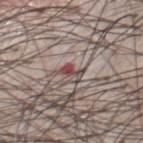Assessment: Part of a total-body skin-imaging series; this lesion was reviewed on a skin check and was not flagged for biopsy. Background: Measured at roughly 2.5 mm in maximum diameter. The lesion is located on the chest. A lesion tile, about 15 mm wide, cut from a 3D total-body photograph. The tile uses white-light illumination. The patient is a male in their 60s. An algorithmic analysis of the crop reported a lesion color around L≈46 a*≈21 b*≈18 in CIELAB, about 13 CIELAB-L* units darker than the surrounding skin, and a normalized lesion–skin contrast near 9.5. The analysis additionally found an automated nevus-likeness rating near 15 out of 100 and a lesion-detection confidence of about 85/100.